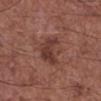Q: Was this lesion biopsied?
A: no biopsy performed (imaged during a skin exam)
Q: What kind of image is this?
A: ~15 mm crop, total-body skin-cancer survey
Q: What is the lesion's diameter?
A: about 4 mm
Q: Patient demographics?
A: male, in their mid-60s
Q: What did automated image analysis measure?
A: an outline eccentricity of about 0.2 (0 = round, 1 = elongated) and a shape-asymmetry score of about 0.3 (0 = symmetric); an average lesion color of about L≈38 a*≈22 b*≈24 (CIELAB) and about 8 CIELAB-L* units darker than the surrounding skin; a within-lesion color-variation index near 4.5/10
Q: How was the tile lit?
A: white-light illumination
Q: Where on the body is the lesion?
A: the right lower leg